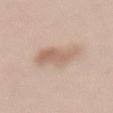| feature | finding |
|---|---|
| notes | imaged on a skin check; not biopsied |
| location | the lower back |
| image | ~15 mm crop, total-body skin-cancer survey |
| subject | female, aged 38 to 42 |